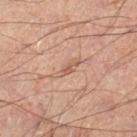Clinical impression: This lesion was catalogued during total-body skin photography and was not selected for biopsy. Background: A 15 mm close-up extracted from a 3D total-body photography capture. Captured under cross-polarized illumination. A male subject aged around 50. Longest diameter approximately 3.5 mm. The lesion is located on the right lower leg.